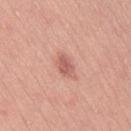Q: Is there a histopathology result?
A: no biopsy performed (imaged during a skin exam)
Q: Automated lesion metrics?
A: a lesion area of about 4 mm², an outline eccentricity of about 0.75 (0 = round, 1 = elongated), and a symmetry-axis asymmetry near 0.15; an automated nevus-likeness rating near 65 out of 100 and a lesion-detection confidence of about 100/100
Q: What kind of image is this?
A: 15 mm crop, total-body photography
Q: How large is the lesion?
A: ≈2.5 mm
Q: Where on the body is the lesion?
A: the right thigh
Q: Who is the patient?
A: female, aged 33 to 37